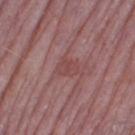Imaged during a routine full-body skin examination; the lesion was not biopsied and no histopathology is available. Captured under white-light illumination. A 15 mm crop from a total-body photograph taken for skin-cancer surveillance. A male subject approximately 75 years of age. Located on the right thigh. About 2.5 mm across.